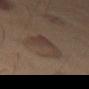Notes:
- notes: imaged on a skin check; not biopsied
- body site: the right thigh
- lesion size: about 4.5 mm
- subject: male, aged 53 to 57
- tile lighting: cross-polarized
- imaging modality: total-body-photography crop, ~15 mm field of view